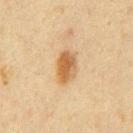biopsy status: imaged on a skin check; not biopsied | tile lighting: cross-polarized | subject: male, about 60 years old | site: the chest | lesion size: about 3.5 mm | acquisition: ~15 mm tile from a whole-body skin photo | TBP lesion metrics: a lesion area of about 7.5 mm², an eccentricity of roughly 0.8, and a shape-asymmetry score of about 0.15 (0 = symmetric); a border-irregularity index near 1.5/10 and peripheral color asymmetry of about 1.5.